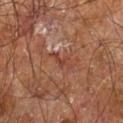Captured during whole-body skin photography for melanoma surveillance; the lesion was not biopsied.
Measured at roughly 3 mm in maximum diameter.
A male patient approximately 60 years of age.
A 15 mm close-up extracted from a 3D total-body photography capture.
This is a cross-polarized tile.
From the right leg.